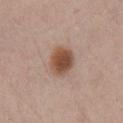Part of a total-body skin-imaging series; this lesion was reviewed on a skin check and was not flagged for biopsy. The lesion is on the chest. This image is a 15 mm lesion crop taken from a total-body photograph. Captured under white-light illumination. Longest diameter approximately 4 mm. The subject is a male aged 33 to 37. The lesion-visualizer software estimated about 13 CIELAB-L* units darker than the surrounding skin and a lesion-to-skin contrast of about 10 (normalized; higher = more distinct). It also reported a border-irregularity rating of about 1/10 and peripheral color asymmetry of about 1. The software also gave an automated nevus-likeness rating near 100 out of 100 and lesion-presence confidence of about 100/100.